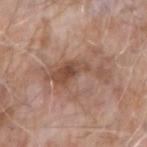biopsy status = no biopsy performed (imaged during a skin exam); site = the right forearm; illumination = white-light illumination; image = ~15 mm crop, total-body skin-cancer survey; patient = male, about 75 years old.